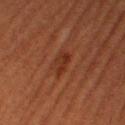Imaged during a routine full-body skin examination; the lesion was not biopsied and no histopathology is available. A female patient aged approximately 55. Located on the right thigh. A lesion tile, about 15 mm wide, cut from a 3D total-body photograph.